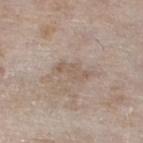Assessment: Imaged during a routine full-body skin examination; the lesion was not biopsied and no histopathology is available. Image and clinical context: Approximately 4 mm at its widest. A female patient aged approximately 75. The tile uses white-light illumination. Located on the left lower leg. A 15 mm close-up extracted from a 3D total-body photography capture. An algorithmic analysis of the crop reported a lesion area of about 6 mm², an eccentricity of roughly 0.9, and a shape-asymmetry score of about 0.4 (0 = symmetric). And it measured a lesion color around L≈57 a*≈13 b*≈25 in CIELAB and a lesion-to-skin contrast of about 5 (normalized; higher = more distinct). And it measured a border-irregularity rating of about 5.5/10, a color-variation rating of about 1/10, and radial color variation of about 0.